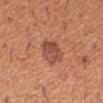workup — total-body-photography surveillance lesion; no biopsy
patient — male, roughly 40 years of age
acquisition — 15 mm crop, total-body photography
site — the right forearm